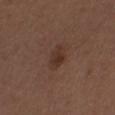location — the right upper arm
subject — male, aged approximately 30
image-analysis metrics — an average lesion color of about L≈32 a*≈18 b*≈24 (CIELAB), roughly 7 lightness units darker than nearby skin, and a normalized lesion–skin contrast near 6.5; a border-irregularity rating of about 2/10, a color-variation rating of about 3/10, and radial color variation of about 1
lighting — white-light illumination
size — about 3.5 mm
imaging modality — ~15 mm crop, total-body skin-cancer survey